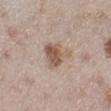Imaged during a routine full-body skin examination; the lesion was not biopsied and no histopathology is available. The recorded lesion diameter is about 4 mm. A female patient, aged 48 to 52. Located on the left lower leg. An algorithmic analysis of the crop reported a nevus-likeness score of about 85/100 and a detector confidence of about 100 out of 100 that the crop contains a lesion. This image is a 15 mm lesion crop taken from a total-body photograph. The tile uses white-light illumination.